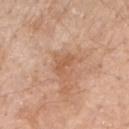  biopsy_status: not biopsied; imaged during a skin examination